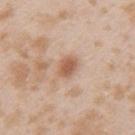Recorded during total-body skin imaging; not selected for excision or biopsy. A roughly 15 mm field-of-view crop from a total-body skin photograph. A female subject, in their mid- to late 20s. This is a white-light tile. About 2.5 mm across. Located on the left upper arm.